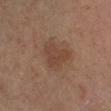Impression: Recorded during total-body skin imaging; not selected for excision or biopsy. Clinical summary: A male subject, approximately 60 years of age. An algorithmic analysis of the crop reported a border-irregularity index near 4/10, a within-lesion color-variation index near 2/10, and radial color variation of about 0.5. And it measured a lesion-detection confidence of about 100/100. On the leg. A roughly 15 mm field-of-view crop from a total-body skin photograph. This is a cross-polarized tile.